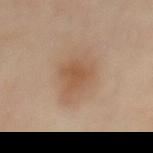  biopsy_status: not biopsied; imaged during a skin examination
  site: front of the torso
  image:
    source: total-body photography crop
    field_of_view_mm: 15